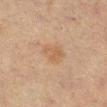Findings:
- notes · no biopsy performed (imaged during a skin exam)
- patient · female, aged approximately 55
- body site · the right lower leg
- diameter · ≈3.5 mm
- lighting · cross-polarized
- image · ~15 mm crop, total-body skin-cancer survey